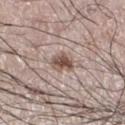Q: Was this lesion biopsied?
A: imaged on a skin check; not biopsied
Q: Where on the body is the lesion?
A: the leg
Q: Patient demographics?
A: male, in their 70s
Q: How large is the lesion?
A: about 3 mm
Q: How was the tile lit?
A: white-light illumination
Q: What kind of image is this?
A: ~15 mm tile from a whole-body skin photo
Q: Automated lesion metrics?
A: a within-lesion color-variation index near 4/10 and a peripheral color-asymmetry measure near 1.5